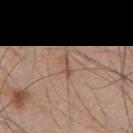{
  "biopsy_status": "not biopsied; imaged during a skin examination",
  "patient": {
    "sex": "male",
    "age_approx": 55
  },
  "automated_metrics": {
    "area_mm2_approx": 2.5,
    "eccentricity": 0.95,
    "shape_asymmetry": 0.45,
    "nevus_likeness_0_100": 0,
    "lesion_detection_confidence_0_100": 55
  },
  "site": "back",
  "lesion_size": {
    "long_diameter_mm_approx": 3.0
  },
  "lighting": "white-light",
  "image": {
    "source": "total-body photography crop",
    "field_of_view_mm": 15
  }
}